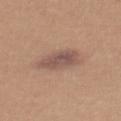Clinical impression:
This lesion was catalogued during total-body skin photography and was not selected for biopsy.
Background:
The total-body-photography lesion software estimated an average lesion color of about L≈52 a*≈18 b*≈24 (CIELAB), a lesion–skin lightness drop of about 10, and a normalized lesion–skin contrast near 8. The software also gave border irregularity of about 2.5 on a 0–10 scale, a color-variation rating of about 3.5/10, and radial color variation of about 1. The software also gave an automated nevus-likeness rating near 30 out of 100 and a detector confidence of about 100 out of 100 that the crop contains a lesion. Approximately 5 mm at its widest. From the upper back. Captured under white-light illumination. A lesion tile, about 15 mm wide, cut from a 3D total-body photograph. A female subject roughly 40 years of age.